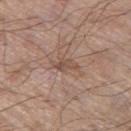Impression: Imaged during a routine full-body skin examination; the lesion was not biopsied and no histopathology is available. Image and clinical context: A male subject, aged 68 to 72. A lesion tile, about 15 mm wide, cut from a 3D total-body photograph. The lesion-visualizer software estimated a footprint of about 3.5 mm², a shape eccentricity near 0.85, and a shape-asymmetry score of about 0.35 (0 = symmetric). And it measured an average lesion color of about L≈50 a*≈18 b*≈25 (CIELAB) and a normalized lesion–skin contrast near 6. The software also gave a border-irregularity rating of about 4/10 and peripheral color asymmetry of about 1. The recorded lesion diameter is about 3 mm. Captured under white-light illumination. The lesion is on the leg.